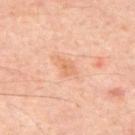No biopsy was performed on this lesion — it was imaged during a full skin examination and was not determined to be concerning. Measured at roughly 2.5 mm in maximum diameter. A 15 mm close-up tile from a total-body photography series done for melanoma screening. The lesion is on the mid back. A male subject, aged 63–67. The lesion-visualizer software estimated an eccentricity of roughly 0.8. Captured under cross-polarized illumination.